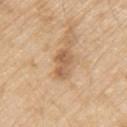Captured during whole-body skin photography for melanoma surveillance; the lesion was not biopsied. Approximately 3.5 mm at its widest. The total-body-photography lesion software estimated a lesion color around L≈59 a*≈19 b*≈35 in CIELAB. And it measured a color-variation rating of about 3/10 and peripheral color asymmetry of about 1. And it measured an automated nevus-likeness rating near 0 out of 100 and a detector confidence of about 100 out of 100 that the crop contains a lesion. The lesion is on the arm. The tile uses white-light illumination. A close-up tile cropped from a whole-body skin photograph, about 15 mm across. The patient is a male approximately 70 years of age.